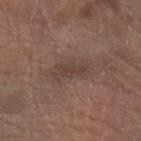The lesion was photographed on a routine skin check and not biopsied; there is no pathology result. A male patient aged approximately 65. A roughly 15 mm field-of-view crop from a total-body skin photograph. The lesion is on the left forearm. The tile uses white-light illumination. Longest diameter approximately 4 mm. Automated tile analysis of the lesion measured a border-irregularity rating of about 6.5/10 and a color-variation rating of about 1/10.